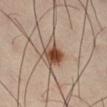A male subject aged 38–42.
Automated tile analysis of the lesion measured a footprint of about 8 mm² and a shape-asymmetry score of about 0.3 (0 = symmetric). The software also gave a border-irregularity rating of about 3/10, a within-lesion color-variation index near 9/10, and peripheral color asymmetry of about 3. And it measured an automated nevus-likeness rating near 100 out of 100 and lesion-presence confidence of about 100/100.
The lesion is on the right thigh.
Cropped from a total-body skin-imaging series; the visible field is about 15 mm.
Longest diameter approximately 4 mm.